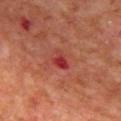Located on the back. A male subject, aged 68 to 72. The tile uses cross-polarized illumination. Measured at roughly 2.5 mm in maximum diameter. A 15 mm close-up extracted from a 3D total-body photography capture.